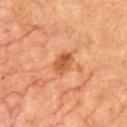workup — imaged on a skin check; not biopsied
imaging modality — 15 mm crop, total-body photography
patient — male, aged approximately 65
anatomic site — the chest